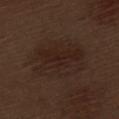follow-up = total-body-photography surveillance lesion; no biopsy
image = 15 mm crop, total-body photography
body site = the left thigh
subject = male, aged 68–72
automated lesion analysis = a lesion color around L≈22 a*≈15 b*≈20 in CIELAB and about 5 CIELAB-L* units darker than the surrounding skin